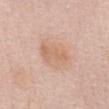This lesion was catalogued during total-body skin photography and was not selected for biopsy. Cropped from a total-body skin-imaging series; the visible field is about 15 mm. Longest diameter approximately 4.5 mm. Located on the front of the torso. A female patient about 60 years old. Imaged with white-light lighting.